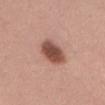biopsy status: total-body-photography surveillance lesion; no biopsy
acquisition: total-body-photography crop, ~15 mm field of view
location: the abdomen
size: ≈4.5 mm
automated lesion analysis: a lesion area of about 10 mm², a shape eccentricity near 0.75, and a shape-asymmetry score of about 0.15 (0 = symmetric); a mean CIELAB color near L≈50 a*≈23 b*≈26 and roughly 15 lightness units darker than nearby skin; border irregularity of about 1.5 on a 0–10 scale, internal color variation of about 4.5 on a 0–10 scale, and peripheral color asymmetry of about 1.5; a nevus-likeness score of about 95/100 and a lesion-detection confidence of about 100/100
tile lighting: white-light illumination
subject: female, in their mid-50s